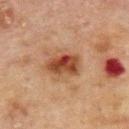{
  "biopsy_status": "not biopsied; imaged during a skin examination",
  "patient": {
    "sex": "male",
    "age_approx": 70
  },
  "image": {
    "source": "total-body photography crop",
    "field_of_view_mm": 15
  },
  "site": "upper back",
  "lighting": "cross-polarized"
}